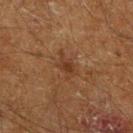Imaged during a routine full-body skin examination; the lesion was not biopsied and no histopathology is available.
Captured under cross-polarized illumination.
Located on the right lower leg.
A male patient aged 58 to 62.
Longest diameter approximately 3 mm.
An algorithmic analysis of the crop reported a lesion area of about 4.5 mm² and two-axis asymmetry of about 0.35. The software also gave roughly 6 lightness units darker than nearby skin and a normalized border contrast of about 6. And it measured a nevus-likeness score of about 0/100 and a lesion-detection confidence of about 100/100.
A lesion tile, about 15 mm wide, cut from a 3D total-body photograph.